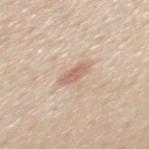Part of a total-body skin-imaging series; this lesion was reviewed on a skin check and was not flagged for biopsy. On the mid back. A roughly 15 mm field-of-view crop from a total-body skin photograph. The lesion's longest dimension is about 3 mm. A male patient about 60 years old. This is a white-light tile.